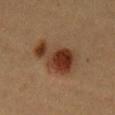biopsy_status: not biopsied; imaged during a skin examination
site: front of the torso
image:
  source: total-body photography crop
  field_of_view_mm: 15
lighting: cross-polarized
patient:
  sex: female
  age_approx: 40
automated_metrics:
  peripheral_color_asymmetry: 2.5